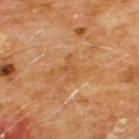{
  "biopsy_status": "not biopsied; imaged during a skin examination",
  "lighting": "cross-polarized",
  "lesion_size": {
    "long_diameter_mm_approx": 3.0
  },
  "image": {
    "source": "total-body photography crop",
    "field_of_view_mm": 15
  },
  "site": "chest",
  "patient": {
    "sex": "male",
    "age_approx": 60
  }
}